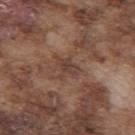Assessment: No biopsy was performed on this lesion — it was imaged during a full skin examination and was not determined to be concerning. Context: A roughly 15 mm field-of-view crop from a total-body skin photograph. A male subject about 75 years old. From the mid back. Longest diameter approximately 2.5 mm.